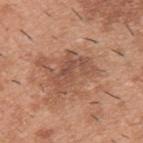biopsy_status: not biopsied; imaged during a skin examination
automated_metrics:
  nevus_likeness_0_100: 0
  lesion_detection_confidence_0_100: 100
image:
  source: total-body photography crop
  field_of_view_mm: 15
site: back
patient:
  sex: male
  age_approx: 40
lighting: white-light
lesion_size:
  long_diameter_mm_approx: 5.0